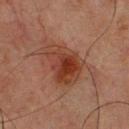Notes:
• notes · no biopsy performed (imaged during a skin exam)
• lighting · cross-polarized
• size · ≈5.5 mm
• location · the upper back
• subject · male, approximately 65 years of age
• automated lesion analysis · a mean CIELAB color near L≈31 a*≈21 b*≈25 and a lesion-to-skin contrast of about 8.5 (normalized; higher = more distinct); a border-irregularity index near 3/10, a color-variation rating of about 6/10, and radial color variation of about 2; lesion-presence confidence of about 100/100
• image source · 15 mm crop, total-body photography